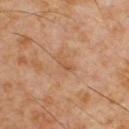– notes: no biopsy performed (imaged during a skin exam)
– acquisition: 15 mm crop, total-body photography
– tile lighting: cross-polarized illumination
– body site: the chest
– patient: male, aged around 60